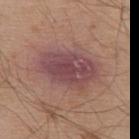Notes:
* biopsy status · total-body-photography surveillance lesion; no biopsy
* acquisition · ~15 mm tile from a whole-body skin photo
* subject · male, aged 53–57
* diameter · ~7.5 mm (longest diameter)
* anatomic site · the back
* lighting · white-light illumination